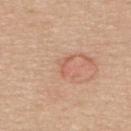Q: Is there a histopathology result?
A: catalogued during a skin exam; not biopsied
Q: Where on the body is the lesion?
A: the upper back
Q: Illumination type?
A: white-light illumination
Q: How was this image acquired?
A: total-body-photography crop, ~15 mm field of view
Q: Who is the patient?
A: female, in their 40s
Q: What did automated image analysis measure?
A: a lesion color around L≈60 a*≈24 b*≈32 in CIELAB and a lesion–skin lightness drop of about 7; a within-lesion color-variation index near 0/10 and radial color variation of about 0; a nevus-likeness score of about 100/100 and a detector confidence of about 100 out of 100 that the crop contains a lesion
Q: How large is the lesion?
A: ≈3 mm